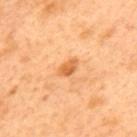Impression:
This lesion was catalogued during total-body skin photography and was not selected for biopsy.
Image and clinical context:
Captured under cross-polarized illumination. An algorithmic analysis of the crop reported a lesion color around L≈64 a*≈29 b*≈47 in CIELAB, roughly 12 lightness units darker than nearby skin, and a normalized border contrast of about 7.5. It also reported an automated nevus-likeness rating near 65 out of 100 and lesion-presence confidence of about 100/100. Located on the mid back. A close-up tile cropped from a whole-body skin photograph, about 15 mm across. A male subject about 50 years old. The lesion's longest dimension is about 2.5 mm.